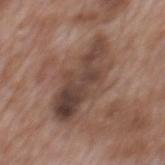workup — imaged on a skin check; not biopsied | body site — the back | patient — male, aged around 70 | acquisition — 15 mm crop, total-body photography.